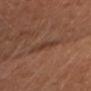{"biopsy_status": "not biopsied; imaged during a skin examination", "site": "head or neck", "automated_metrics": {"border_irregularity_0_10": 4.0, "peripheral_color_asymmetry": 0.0}, "image": {"source": "total-body photography crop", "field_of_view_mm": 15}, "patient": {"sex": "female", "age_approx": 40}}